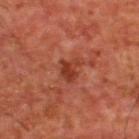  biopsy_status: not biopsied; imaged during a skin examination
  site: upper back
  patient:
    sex: male
    age_approx: 65
  automated_metrics:
    area_mm2_approx: 5.0
    eccentricity: 0.6
    shape_asymmetry: 0.4
  image:
    source: total-body photography crop
    field_of_view_mm: 15